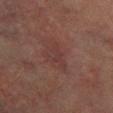Case summary:
• location: the leg
• image source: ~15 mm crop, total-body skin-cancer survey
• diameter: ~3.5 mm (longest diameter)
• lighting: cross-polarized
• subject: male, aged 73 to 77